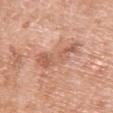This lesion was catalogued during total-body skin photography and was not selected for biopsy.
A 15 mm close-up extracted from a 3D total-body photography capture.
An algorithmic analysis of the crop reported a lesion area of about 10 mm², an outline eccentricity of about 0.9 (0 = round, 1 = elongated), and a symmetry-axis asymmetry near 0.4. The analysis additionally found roughly 9 lightness units darker than nearby skin and a normalized lesion–skin contrast near 6. It also reported a classifier nevus-likeness of about 0/100 and a lesion-detection confidence of about 100/100.
A female subject, aged approximately 60.
About 5.5 mm across.
The lesion is on the front of the torso.